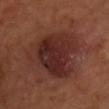image source: ~15 mm tile from a whole-body skin photo; subject: female, aged 38–42; site: the chest; pathology: a seborrheic keratosis.